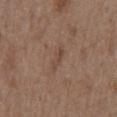workup: no biopsy performed (imaged during a skin exam); subject: male, approximately 50 years of age; image source: ~15 mm tile from a whole-body skin photo; anatomic site: the back.